The total-body-photography lesion software estimated a lesion area of about 2.5 mm², a shape eccentricity near 0.45, and a symmetry-axis asymmetry near 0.3. The software also gave about 12 CIELAB-L* units darker than the surrounding skin and a normalized lesion–skin contrast near 9. The analysis additionally found a peripheral color-asymmetry measure near 0.5. The analysis additionally found an automated nevus-likeness rating near 95 out of 100 and a lesion-detection confidence of about 100/100. Located on the front of the torso. A 15 mm crop from a total-body photograph taken for skin-cancer surveillance. Longest diameter approximately 2 mm. The tile uses cross-polarized illumination. A subject aged approximately 60 — 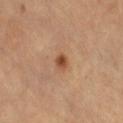Diagnosis:
The lesion was biopsied, and histopathology showed a melanocytic nevus (benign).